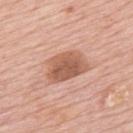Part of a total-body skin-imaging series; this lesion was reviewed on a skin check and was not flagged for biopsy.
Cropped from a whole-body photographic skin survey; the tile spans about 15 mm.
On the upper back.
A male patient, roughly 80 years of age.
The lesion's longest dimension is about 5 mm.